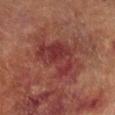<tbp_lesion>
  <biopsy_status>not biopsied; imaged during a skin examination</biopsy_status>
  <site>leg</site>
  <image>
    <source>total-body photography crop</source>
    <field_of_view_mm>15</field_of_view_mm>
  </image>
  <patient>
    <sex>male</sex>
    <age_approx>70</age_approx>
  </patient>
</tbp_lesion>